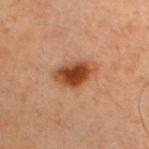– workup — no biopsy performed (imaged during a skin exam)
– acquisition — total-body-photography crop, ~15 mm field of view
– image-analysis metrics — an average lesion color of about L≈35 a*≈20 b*≈29 (CIELAB), roughly 12 lightness units darker than nearby skin, and a normalized lesion–skin contrast near 10.5; a classifier nevus-likeness of about 100/100 and a lesion-detection confidence of about 100/100
– anatomic site — the chest
– subject — male, in their 60s
– diameter — about 5 mm